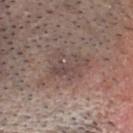workup — no biopsy performed (imaged during a skin exam)
patient — male, approximately 65 years of age
site — the head or neck
diameter — about 6 mm
TBP lesion metrics — a mean CIELAB color near L≈47 a*≈15 b*≈20, roughly 7 lightness units darker than nearby skin, and a normalized border contrast of about 5.5
acquisition — ~15 mm tile from a whole-body skin photo
illumination — white-light illumination